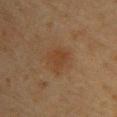Imaged during a routine full-body skin examination; the lesion was not biopsied and no histopathology is available.
A female subject, aged around 45.
The lesion is on the upper back.
A region of skin cropped from a whole-body photographic capture, roughly 15 mm wide.
The recorded lesion diameter is about 2.5 mm.